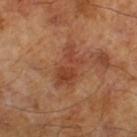No biopsy was performed on this lesion — it was imaged during a full skin examination and was not determined to be concerning.
A male patient in their mid-60s.
A roughly 15 mm field-of-view crop from a total-body skin photograph.
Captured under cross-polarized illumination.